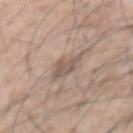Context: A 15 mm crop from a total-body photograph taken for skin-cancer surveillance. A male subject roughly 55 years of age. About 4 mm across. Imaged with white-light lighting. On the chest.